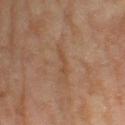Recorded during total-body skin imaging; not selected for excision or biopsy.
A region of skin cropped from a whole-body photographic capture, roughly 15 mm wide.
A female subject approximately 60 years of age.
Imaged with cross-polarized lighting.
The recorded lesion diameter is about 3 mm.
On the leg.